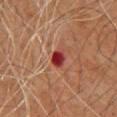Clinical impression:
Captured during whole-body skin photography for melanoma surveillance; the lesion was not biopsied.
Background:
The lesion is on the chest. A male subject, roughly 65 years of age. This image is a 15 mm lesion crop taken from a total-body photograph.